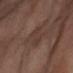follow-up = catalogued during a skin exam; not biopsied
subject = female, aged 53–57
size = ~4.5 mm (longest diameter)
imaging modality = ~15 mm crop, total-body skin-cancer survey
site = the abdomen
lighting = cross-polarized illumination